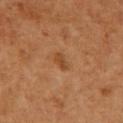Impression:
No biopsy was performed on this lesion — it was imaged during a full skin examination and was not determined to be concerning.